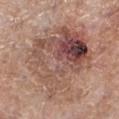This lesion was catalogued during total-body skin photography and was not selected for biopsy. A region of skin cropped from a whole-body photographic capture, roughly 15 mm wide. Captured under white-light illumination. The lesion is located on the left lower leg. Approximately 9.5 mm at its widest. A female patient, about 75 years old.